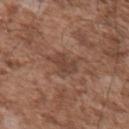{
  "biopsy_status": "not biopsied; imaged during a skin examination",
  "patient": {
    "sex": "male",
    "age_approx": 55
  },
  "image": {
    "source": "total-body photography crop",
    "field_of_view_mm": 15
  },
  "lesion_size": {
    "long_diameter_mm_approx": 3.5
  },
  "site": "upper back"
}